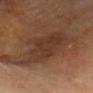This is a cross-polarized tile. A male subject, roughly 60 years of age. The lesion-visualizer software estimated two-axis asymmetry of about 0.2. It also reported an average lesion color of about L≈34 a*≈18 b*≈26 (CIELAB) and a normalized border contrast of about 7. The analysis additionally found a nevus-likeness score of about 0/100. From the head or neck. About 7.5 mm across. A region of skin cropped from a whole-body photographic capture, roughly 15 mm wide.